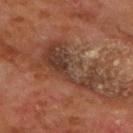No biopsy was performed on this lesion — it was imaged during a full skin examination and was not determined to be concerning. This is a cross-polarized tile. The lesion is located on the chest. A roughly 15 mm field-of-view crop from a total-body skin photograph. Approximately 9 mm at its widest. A male patient aged 58–62. The lesion-visualizer software estimated an area of roughly 22 mm², a shape eccentricity near 0.9, and a shape-asymmetry score of about 0.65 (0 = symmetric). And it measured lesion-presence confidence of about 95/100.